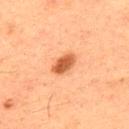Q: Was a biopsy performed?
A: no biopsy performed (imaged during a skin exam)
Q: How was this image acquired?
A: 15 mm crop, total-body photography
Q: Patient demographics?
A: male, approximately 50 years of age
Q: Where on the body is the lesion?
A: the upper back